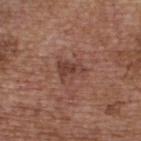Captured during whole-body skin photography for melanoma surveillance; the lesion was not biopsied.
A female patient, in their mid- to late 60s.
Automated image analysis of the tile measured a footprint of about 6.5 mm². It also reported a border-irregularity rating of about 4.5/10, internal color variation of about 4 on a 0–10 scale, and a peripheral color-asymmetry measure near 2. The software also gave a nevus-likeness score of about 0/100 and a lesion-detection confidence of about 100/100.
On the upper back.
A roughly 15 mm field-of-view crop from a total-body skin photograph.
Imaged with white-light lighting.
Longest diameter approximately 3.5 mm.